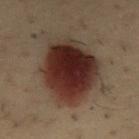<tbp_lesion>
<biopsy_status>not biopsied; imaged during a skin examination</biopsy_status>
</tbp_lesion>